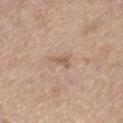follow-up: no biopsy performed (imaged during a skin exam)
subject: male, in their 70s
imaging modality: total-body-photography crop, ~15 mm field of view
lesion size: about 2.5 mm
location: the left thigh
automated lesion analysis: a shape eccentricity near 0.85 and a shape-asymmetry score of about 0.55 (0 = symmetric); a mean CIELAB color near L≈58 a*≈17 b*≈30, a lesion–skin lightness drop of about 8, and a normalized lesion–skin contrast near 6; an automated nevus-likeness rating near 0 out of 100 and a detector confidence of about 100 out of 100 that the crop contains a lesion